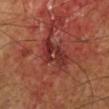Captured during whole-body skin photography for melanoma surveillance; the lesion was not biopsied.
The lesion is on the left lower leg.
A male patient in their 60s.
A roughly 15 mm field-of-view crop from a total-body skin photograph.